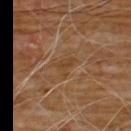Image and clinical context:
Measured at roughly 2.5 mm in maximum diameter. The lesion is on the chest. Captured under cross-polarized illumination. A male subject, aged around 60. The total-body-photography lesion software estimated a shape eccentricity near 0.7 and two-axis asymmetry of about 0.4. And it measured a mean CIELAB color near L≈40 a*≈18 b*≈32, about 6 CIELAB-L* units darker than the surrounding skin, and a normalized border contrast of about 5.5. This image is a 15 mm lesion crop taken from a total-body photograph.